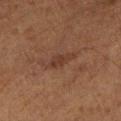The lesion was tiled from a total-body skin photograph and was not biopsied. The subject is a male roughly 75 years of age. A roughly 15 mm field-of-view crop from a total-body skin photograph. The lesion is on the left lower leg. Captured under cross-polarized illumination. An algorithmic analysis of the crop reported an area of roughly 4 mm², an eccentricity of roughly 0.9, and a symmetry-axis asymmetry near 0.25. The software also gave a mean CIELAB color near L≈29 a*≈17 b*≈23 and about 6 CIELAB-L* units darker than the surrounding skin. And it measured an automated nevus-likeness rating near 5 out of 100 and a detector confidence of about 95 out of 100 that the crop contains a lesion.